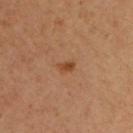Imaged during a routine full-body skin examination; the lesion was not biopsied and no histopathology is available. Approximately 2 mm at its widest. A roughly 15 mm field-of-view crop from a total-body skin photograph. An algorithmic analysis of the crop reported roughly 9 lightness units darker than nearby skin and a lesion-to-skin contrast of about 7.5 (normalized; higher = more distinct). It also reported a nevus-likeness score of about 75/100 and a lesion-detection confidence of about 100/100. The patient is a male about 65 years old. The lesion is on the chest. The tile uses cross-polarized illumination.